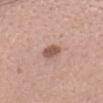The lesion was photographed on a routine skin check and not biopsied; there is no pathology result. Automated image analysis of the tile measured a footprint of about 4.5 mm², an eccentricity of roughly 0.6, and a shape-asymmetry score of about 0.15 (0 = symmetric). The analysis additionally found a border-irregularity index near 1.5/10 and peripheral color asymmetry of about 1. The software also gave a nevus-likeness score of about 40/100. A 15 mm crop from a total-body photograph taken for skin-cancer surveillance. A female patient, in their mid- to late 20s. On the head or neck. Approximately 2.5 mm at its widest.